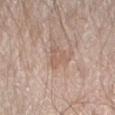Q: Is there a histopathology result?
A: catalogued during a skin exam; not biopsied
Q: How was this image acquired?
A: ~15 mm crop, total-body skin-cancer survey
Q: Patient demographics?
A: male, aged 78 to 82
Q: Lesion location?
A: the right forearm